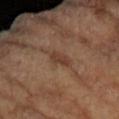notes: total-body-photography surveillance lesion; no biopsy | image-analysis metrics: a mean CIELAB color near L≈39 a*≈19 b*≈27 and a lesion-to-skin contrast of about 7 (normalized; higher = more distinct); a border-irregularity rating of about 3/10, a within-lesion color-variation index near 1/10, and peripheral color asymmetry of about 0 | image source: total-body-photography crop, ~15 mm field of view | patient: female, in their 70s | location: the arm | lighting: cross-polarized illumination.